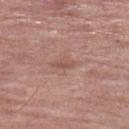Recorded during total-body skin imaging; not selected for excision or biopsy. Cropped from a total-body skin-imaging series; the visible field is about 15 mm. A female patient about 85 years old or older. The lesion is located on the leg.